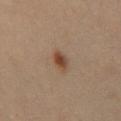<case>
<biopsy_status>not biopsied; imaged during a skin examination</biopsy_status>
<image>
  <source>total-body photography crop</source>
  <field_of_view_mm>15</field_of_view_mm>
</image>
<patient>
  <sex>male</sex>
  <age_approx>65</age_approx>
</patient>
<site>chest</site>
</case>